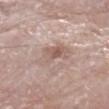<lesion>
<biopsy_status>not biopsied; imaged during a skin examination</biopsy_status>
<automated_metrics>
  <cielab_L>59</cielab_L>
  <cielab_a>16</cielab_a>
  <cielab_b>23</cielab_b>
  <vs_skin_darker_L>9.0</vs_skin_darker_L>
  <border_irregularity_0_10>4.5</border_irregularity_0_10>
  <color_variation_0_10>5.5</color_variation_0_10>
  <lesion_detection_confidence_0_100>100</lesion_detection_confidence_0_100>
</automated_metrics>
<image>
  <source>total-body photography crop</source>
  <field_of_view_mm>15</field_of_view_mm>
</image>
<patient>
  <sex>male</sex>
  <age_approx>80</age_approx>
</patient>
<lesion_size>
  <long_diameter_mm_approx>5.0</long_diameter_mm_approx>
</lesion_size>
<site>leg</site>
</lesion>